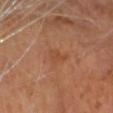No biopsy was performed on this lesion — it was imaged during a full skin examination and was not determined to be concerning.
This image is a 15 mm lesion crop taken from a total-body photograph.
The lesion-visualizer software estimated a lesion area of about 3 mm², an outline eccentricity of about 0.9 (0 = round, 1 = elongated), and a shape-asymmetry score of about 0.4 (0 = symmetric). The analysis additionally found a classifier nevus-likeness of about 0/100 and a detector confidence of about 100 out of 100 that the crop contains a lesion.
The subject is a male in their mid- to late 60s.
The lesion's longest dimension is about 3 mm.